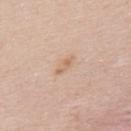Clinical impression: Part of a total-body skin-imaging series; this lesion was reviewed on a skin check and was not flagged for biopsy. Background: A lesion tile, about 15 mm wide, cut from a 3D total-body photograph. Captured under white-light illumination. Automated image analysis of the tile measured a mean CIELAB color near L≈65 a*≈17 b*≈32, a lesion–skin lightness drop of about 7, and a normalized border contrast of about 5.5. The software also gave an automated nevus-likeness rating near 0 out of 100 and lesion-presence confidence of about 100/100. The subject is a male approximately 40 years of age. The lesion is on the upper back.